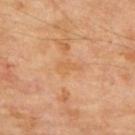Clinical impression:
Recorded during total-body skin imaging; not selected for excision or biopsy.
Image and clinical context:
A male patient aged 68–72. Cropped from a whole-body photographic skin survey; the tile spans about 15 mm. Located on the back.